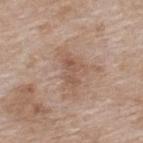The lesion was tiled from a total-body skin photograph and was not biopsied. A 15 mm close-up tile from a total-body photography series done for melanoma screening. The lesion is located on the upper back. A female patient in their mid-70s. Approximately 3 mm at its widest.